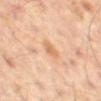Assessment:
This lesion was catalogued during total-body skin photography and was not selected for biopsy.
Clinical summary:
About 2.5 mm across. Captured under cross-polarized illumination. A 15 mm close-up extracted from a 3D total-body photography capture. The lesion is located on the mid back. The total-body-photography lesion software estimated a lesion area of about 3 mm² and a shape-asymmetry score of about 0.3 (0 = symmetric). It also reported a lesion color around L≈62 a*≈21 b*≈35 in CIELAB and a normalized lesion–skin contrast near 6. The software also gave border irregularity of about 3 on a 0–10 scale, internal color variation of about 1.5 on a 0–10 scale, and a peripheral color-asymmetry measure near 0.5. A male subject, aged around 60.